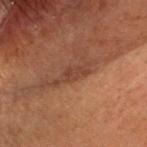The lesion was tiled from a total-body skin photograph and was not biopsied. A male subject roughly 55 years of age. A 15 mm close-up tile from a total-body photography series done for melanoma screening. On the head or neck. This is a cross-polarized tile. Longest diameter approximately 2.5 mm.